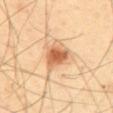Impression: Recorded during total-body skin imaging; not selected for excision or biopsy. Context: Imaged with cross-polarized lighting. Automated image analysis of the tile measured a footprint of about 7 mm² and a symmetry-axis asymmetry near 0.35. The analysis additionally found about 15 CIELAB-L* units darker than the surrounding skin and a normalized lesion–skin contrast near 9.5. The analysis additionally found internal color variation of about 4 on a 0–10 scale. On the upper back. A close-up tile cropped from a whole-body skin photograph, about 15 mm across. The recorded lesion diameter is about 4.5 mm. The subject is a male aged 63 to 67.